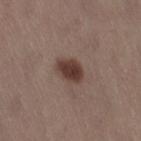Clinical impression:
Recorded during total-body skin imaging; not selected for excision or biopsy.
Clinical summary:
Automated tile analysis of the lesion measured a shape eccentricity near 0.65. It also reported a color-variation rating of about 3/10. Imaged with white-light lighting. About 3.5 mm across. A female subject aged around 50. The lesion is on the right thigh. A 15 mm close-up extracted from a 3D total-body photography capture.